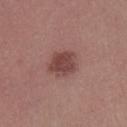Clinical impression: The lesion was photographed on a routine skin check and not biopsied; there is no pathology result. Clinical summary: A 15 mm close-up tile from a total-body photography series done for melanoma screening. Approximately 3.5 mm at its widest. The subject is a male in their mid- to late 40s. On the right lower leg. Imaged with white-light lighting.